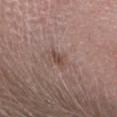| field | value |
|---|---|
| follow-up | total-body-photography surveillance lesion; no biopsy |
| image-analysis metrics | a mean CIELAB color near L≈47 a*≈18 b*≈23 and a normalized lesion–skin contrast near 6.5; a nevus-likeness score of about 0/100 |
| illumination | white-light |
| image | ~15 mm tile from a whole-body skin photo |
| patient | male, about 30 years old |
| diameter | ≈2.5 mm |